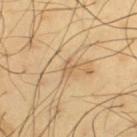Findings:
* workup — catalogued during a skin exam; not biopsied
* location — the left thigh
* image — ~15 mm crop, total-body skin-cancer survey
* subject — male, about 65 years old